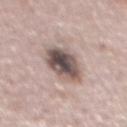– follow-up: total-body-photography surveillance lesion; no biopsy
– acquisition: total-body-photography crop, ~15 mm field of view
– TBP lesion metrics: a within-lesion color-variation index near 9.5/10 and peripheral color asymmetry of about 3
– location: the abdomen
– patient: male, aged approximately 75